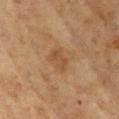{
  "patient": {
    "sex": "female",
    "age_approx": 60
  },
  "site": "front of the torso",
  "image": {
    "source": "total-body photography crop",
    "field_of_view_mm": 15
  }
}